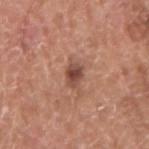biopsy status: catalogued during a skin exam; not biopsied | site: the arm | image source: ~15 mm crop, total-body skin-cancer survey | automated metrics: a mean CIELAB color near L≈47 a*≈23 b*≈27, a lesion–skin lightness drop of about 12, and a lesion-to-skin contrast of about 8.5 (normalized; higher = more distinct) | illumination: white-light illumination | patient: male, aged around 65 | diameter: ~2.5 mm (longest diameter).